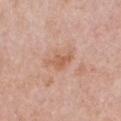Captured during whole-body skin photography for melanoma surveillance; the lesion was not biopsied. A female subject, approximately 50 years of age. The tile uses white-light illumination. Automated image analysis of the tile measured a lesion area of about 5.5 mm² and an outline eccentricity of about 0.8 (0 = round, 1 = elongated). It also reported a border-irregularity rating of about 4/10, a within-lesion color-variation index near 2/10, and a peripheral color-asymmetry measure near 0.5. And it measured a classifier nevus-likeness of about 0/100 and lesion-presence confidence of about 100/100. The lesion is on the chest. About 3.5 mm across. A roughly 15 mm field-of-view crop from a total-body skin photograph.